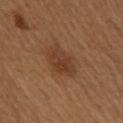No biopsy was performed on this lesion — it was imaged during a full skin examination and was not determined to be concerning.
This is a cross-polarized tile.
Approximately 4 mm at its widest.
Cropped from a total-body skin-imaging series; the visible field is about 15 mm.
The patient is a female approximately 50 years of age.
The lesion is located on the upper back.